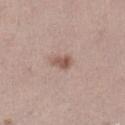This lesion was catalogued during total-body skin photography and was not selected for biopsy. The recorded lesion diameter is about 2.5 mm. The lesion is located on the left lower leg. A 15 mm crop from a total-body photograph taken for skin-cancer surveillance. The subject is a female aged approximately 25. This is a white-light tile.